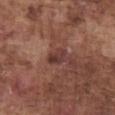No biopsy was performed on this lesion — it was imaged during a full skin examination and was not determined to be concerning. The patient is a male in their mid- to late 70s. The tile uses white-light illumination. The lesion is on the abdomen. Approximately 2.5 mm at its widest. A region of skin cropped from a whole-body photographic capture, roughly 15 mm wide.